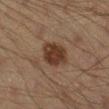workup = no biopsy performed (imaged during a skin exam)
acquisition = total-body-photography crop, ~15 mm field of view
patient = male, roughly 45 years of age
site = the leg